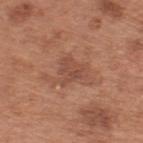Q: Was a biopsy performed?
A: catalogued during a skin exam; not biopsied
Q: What kind of image is this?
A: 15 mm crop, total-body photography
Q: What is the anatomic site?
A: the upper back
Q: How large is the lesion?
A: ≈3.5 mm
Q: How was the tile lit?
A: white-light
Q: What are the patient's age and sex?
A: male, in their mid- to late 60s